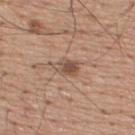Captured during whole-body skin photography for melanoma surveillance; the lesion was not biopsied. A 15 mm close-up tile from a total-body photography series done for melanoma screening. A male patient aged 53 to 57. The lesion is on the upper back. About 3 mm across.